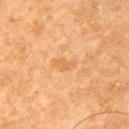biopsy_status: not biopsied; imaged during a skin examination
patient:
  sex: male
  age_approx: 65
image:
  source: total-body photography crop
  field_of_view_mm: 15
site: arm
lesion_size:
  long_diameter_mm_approx: 2.5
automated_metrics:
  area_mm2_approx: 3.5
  eccentricity: 0.85
  shape_asymmetry: 0.4
  cielab_L: 52
  cielab_a: 20
  cielab_b: 37
  vs_skin_darker_L: 5.0
  vs_skin_contrast_norm: 5.0
  border_irregularity_0_10: 4.0
  color_variation_0_10: 1.0
  peripheral_color_asymmetry: 0.0